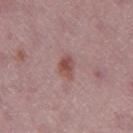workup: total-body-photography surveillance lesion; no biopsy | subject: female, aged approximately 50 | automated metrics: a footprint of about 4.5 mm², a shape eccentricity near 0.75, and a shape-asymmetry score of about 0.3 (0 = symmetric); a mean CIELAB color near L≈50 a*≈22 b*≈23, roughly 9 lightness units darker than nearby skin, and a normalized lesion–skin contrast near 8; a within-lesion color-variation index near 4.5/10 and radial color variation of about 1.5 | tile lighting: white-light | imaging modality: total-body-photography crop, ~15 mm field of view | site: the leg.